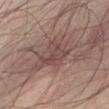Q: Was a biopsy performed?
A: no biopsy performed (imaged during a skin exam)
Q: What lighting was used for the tile?
A: white-light illumination
Q: How was this image acquired?
A: total-body-photography crop, ~15 mm field of view
Q: What are the patient's age and sex?
A: male, aged 53 to 57
Q: Where on the body is the lesion?
A: the front of the torso
Q: What is the lesion's diameter?
A: ≈4 mm